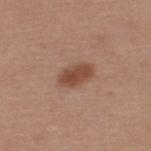Acquisition and patient details: A male patient aged approximately 40. A 15 mm crop from a total-body photograph taken for skin-cancer surveillance. On the upper back.